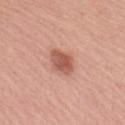<tbp_lesion>
<biopsy_status>not biopsied; imaged during a skin examination</biopsy_status>
<patient>
  <sex>female</sex>
  <age_approx>55</age_approx>
</patient>
<lighting>white-light</lighting>
<lesion_size>
  <long_diameter_mm_approx>3.0</long_diameter_mm_approx>
</lesion_size>
<image>
  <source>total-body photography crop</source>
  <field_of_view_mm>15</field_of_view_mm>
</image>
<site>arm</site>
</tbp_lesion>